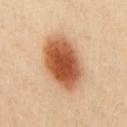Recorded during total-body skin imaging; not selected for excision or biopsy. This image is a 15 mm lesion crop taken from a total-body photograph. Imaged with cross-polarized lighting. The subject is a male about 50 years old. Automated tile analysis of the lesion measured a mean CIELAB color near L≈56 a*≈25 b*≈37, about 18 CIELAB-L* units darker than the surrounding skin, and a normalized border contrast of about 11.5. It also reported border irregularity of about 1.5 on a 0–10 scale, a color-variation rating of about 6.5/10, and radial color variation of about 1.5. And it measured a classifier nevus-likeness of about 100/100 and a detector confidence of about 100 out of 100 that the crop contains a lesion. The lesion's longest dimension is about 7 mm. On the front of the torso.